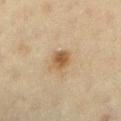No biopsy was performed on this lesion — it was imaged during a full skin examination and was not determined to be concerning. Located on the left lower leg. The subject is a female approximately 65 years of age. Cropped from a total-body skin-imaging series; the visible field is about 15 mm.